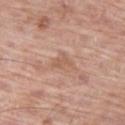{
  "image": {
    "source": "total-body photography crop",
    "field_of_view_mm": 15
  },
  "patient": {
    "sex": "male",
    "age_approx": 70
  },
  "lesion_size": {
    "long_diameter_mm_approx": 3.0
  },
  "site": "left thigh"
}